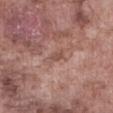Q: Is there a histopathology result?
A: imaged on a skin check; not biopsied
Q: What is the anatomic site?
A: the abdomen
Q: What are the patient's age and sex?
A: male, aged 73–77
Q: Automated lesion metrics?
A: an outline eccentricity of about 0.9 (0 = round, 1 = elongated) and a shape-asymmetry score of about 0.3 (0 = symmetric); a border-irregularity rating of about 4/10 and radial color variation of about 0.5; a classifier nevus-likeness of about 0/100 and a lesion-detection confidence of about 85/100
Q: Lesion size?
A: ~3.5 mm (longest diameter)
Q: What is the imaging modality?
A: total-body-photography crop, ~15 mm field of view